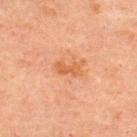Impression:
The lesion was tiled from a total-body skin photograph and was not biopsied.
Acquisition and patient details:
The recorded lesion diameter is about 3 mm. Imaged with cross-polarized lighting. Cropped from a total-body skin-imaging series; the visible field is about 15 mm. Located on the back. A male patient, aged approximately 60. Automated tile analysis of the lesion measured a lesion-to-skin contrast of about 6.5 (normalized; higher = more distinct). And it measured a border-irregularity index near 4/10, a color-variation rating of about 1.5/10, and a peripheral color-asymmetry measure near 0.5.